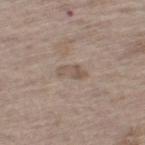| key | value |
|---|---|
| biopsy status | no biopsy performed (imaged during a skin exam) |
| body site | the left thigh |
| tile lighting | white-light illumination |
| lesion diameter | ≈3.5 mm |
| patient | male, roughly 70 years of age |
| acquisition | ~15 mm crop, total-body skin-cancer survey |